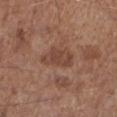notes: no biopsy performed (imaged during a skin exam) | lesion diameter: ≈4.5 mm | automated metrics: an area of roughly 7.5 mm², an eccentricity of roughly 0.85, and a shape-asymmetry score of about 0.35 (0 = symmetric); a mean CIELAB color near L≈44 a*≈21 b*≈27, roughly 8 lightness units darker than nearby skin, and a normalized lesion–skin contrast near 6.5; a border-irregularity rating of about 3.5/10 and a within-lesion color-variation index near 2.5/10 | anatomic site: the right lower leg | acquisition: ~15 mm crop, total-body skin-cancer survey | subject: male, aged 58–62 | lighting: white-light.